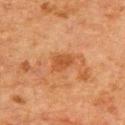Notes:
- workup: total-body-photography surveillance lesion; no biopsy
- anatomic site: the chest
- tile lighting: cross-polarized illumination
- lesion diameter: about 3 mm
- patient: male, approximately 80 years of age
- image source: ~15 mm crop, total-body skin-cancer survey